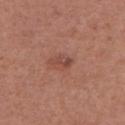Assessment:
Captured during whole-body skin photography for melanoma surveillance; the lesion was not biopsied.
Image and clinical context:
A close-up tile cropped from a whole-body skin photograph, about 15 mm across. The lesion-visualizer software estimated a shape eccentricity near 0.85 and two-axis asymmetry of about 0.45. It also reported an automated nevus-likeness rating near 15 out of 100 and a lesion-detection confidence of about 100/100. A female patient about 50 years old. The lesion is on the chest. The tile uses white-light illumination.